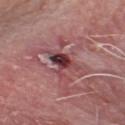The lesion was tiled from a total-body skin photograph and was not biopsied. On the head or neck. This image is a 15 mm lesion crop taken from a total-body photograph. A male patient, roughly 65 years of age. Captured under white-light illumination.